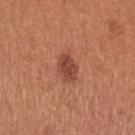Captured during whole-body skin photography for melanoma surveillance; the lesion was not biopsied.
Automated tile analysis of the lesion measured an average lesion color of about L≈46 a*≈26 b*≈31 (CIELAB) and a normalized border contrast of about 8.5. The software also gave a border-irregularity index near 2.5/10 and a color-variation rating of about 2.5/10.
This is a white-light tile.
This image is a 15 mm lesion crop taken from a total-body photograph.
The lesion is located on the left upper arm.
A female patient aged 53–57.
The recorded lesion diameter is about 3 mm.